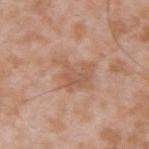workup: no biopsy performed (imaged during a skin exam)
subject: male, in their mid- to late 30s
lighting: white-light illumination
body site: the upper back
size: ≈5 mm
acquisition: ~15 mm crop, total-body skin-cancer survey